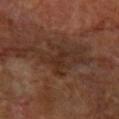Imaged during a routine full-body skin examination; the lesion was not biopsied and no histopathology is available.
The lesion-visualizer software estimated a footprint of about 3 mm² and an eccentricity of roughly 0.9. And it measured a classifier nevus-likeness of about 0/100 and a detector confidence of about 85 out of 100 that the crop contains a lesion.
Approximately 2.5 mm at its widest.
A 15 mm close-up extracted from a 3D total-body photography capture.
Captured under cross-polarized illumination.
The subject is a female in their mid- to late 60s.
The lesion is located on the right forearm.